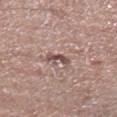Clinical impression: This lesion was catalogued during total-body skin photography and was not selected for biopsy. Acquisition and patient details: The lesion is on the left lower leg. Cropped from a total-body skin-imaging series; the visible field is about 15 mm. Automated tile analysis of the lesion measured an average lesion color of about L≈50 a*≈18 b*≈21 (CIELAB), a lesion–skin lightness drop of about 12, and a normalized lesion–skin contrast near 8.5. Longest diameter approximately 3 mm. Captured under white-light illumination. The patient is a male aged approximately 55.